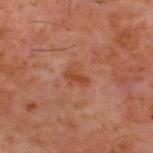patient: male, in their 60s
TBP lesion metrics: an outline eccentricity of about 0.7 (0 = round, 1 = elongated) and a shape-asymmetry score of about 0.35 (0 = symmetric); an automated nevus-likeness rating near 0 out of 100
lesion diameter: ~2.5 mm (longest diameter)
image: 15 mm crop, total-body photography
anatomic site: the upper back
tile lighting: cross-polarized illumination